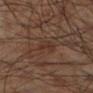Findings:
* notes: total-body-photography surveillance lesion; no biopsy